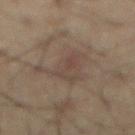workup=no biopsy performed (imaged during a skin exam).